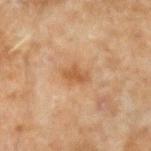– workup · total-body-photography surveillance lesion; no biopsy
– image · 15 mm crop, total-body photography
– location · the left forearm
– subject · male, about 45 years old
– tile lighting · cross-polarized
– diameter · ~3 mm (longest diameter)
– automated metrics · a lesion color around L≈45 a*≈18 b*≈32 in CIELAB; a nevus-likeness score of about 0/100 and a lesion-detection confidence of about 100/100